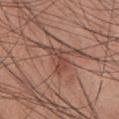{"biopsy_status": "not biopsied; imaged during a skin examination", "lighting": "white-light", "image": {"source": "total-body photography crop", "field_of_view_mm": 15}, "automated_metrics": {"area_mm2_approx": 7.0, "shape_asymmetry": 0.35, "nevus_likeness_0_100": 0, "lesion_detection_confidence_0_100": 95}, "site": "chest", "lesion_size": {"long_diameter_mm_approx": 3.5}, "patient": {"sex": "male", "age_approx": 60}}